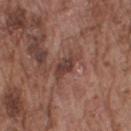Clinical impression: Recorded during total-body skin imaging; not selected for excision or biopsy. Context: A region of skin cropped from a whole-body photographic capture, roughly 15 mm wide. The lesion is on the right upper arm. Approximately 4 mm at its widest. A male patient in their mid- to late 70s. The total-body-photography lesion software estimated a color-variation rating of about 2.5/10 and radial color variation of about 0.5. And it measured a nevus-likeness score of about 0/100 and a lesion-detection confidence of about 90/100. This is a white-light tile.